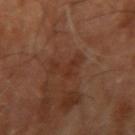{"biopsy_status": "not biopsied; imaged during a skin examination", "patient": {"sex": "male", "age_approx": 70}, "site": "right upper arm", "lighting": "cross-polarized", "automated_metrics": {"eccentricity": 0.85, "shape_asymmetry": 0.8, "nevus_likeness_0_100": 0, "lesion_detection_confidence_0_100": 100}, "image": {"source": "total-body photography crop", "field_of_view_mm": 15}, "lesion_size": {"long_diameter_mm_approx": 4.0}}